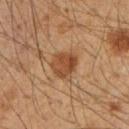{"biopsy_status": "not biopsied; imaged during a skin examination", "patient": {"sex": "male", "age_approx": 50}, "site": "right upper arm", "lesion_size": {"long_diameter_mm_approx": 4.0}, "lighting": "cross-polarized", "image": {"source": "total-body photography crop", "field_of_view_mm": 15}}